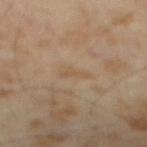Notes:
* notes: no biopsy performed (imaged during a skin exam)
* patient: male, approximately 65 years of age
* lighting: cross-polarized
* TBP lesion metrics: a footprint of about 2.5 mm², an outline eccentricity of about 0.9 (0 = round, 1 = elongated), and a symmetry-axis asymmetry near 0.35; a lesion color around L≈52 a*≈14 b*≈31 in CIELAB and a lesion–skin lightness drop of about 5; border irregularity of about 4 on a 0–10 scale, internal color variation of about 0 on a 0–10 scale, and a peripheral color-asymmetry measure near 0; a nevus-likeness score of about 0/100 and a detector confidence of about 100 out of 100 that the crop contains a lesion
* image source: total-body-photography crop, ~15 mm field of view
* lesion size: ≈3 mm